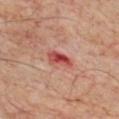Q: Where on the body is the lesion?
A: the chest
Q: Illumination type?
A: cross-polarized
Q: What are the patient's age and sex?
A: male, roughly 70 years of age
Q: What kind of image is this?
A: ~15 mm tile from a whole-body skin photo
Q: What did automated image analysis measure?
A: a footprint of about 5 mm² and a shape-asymmetry score of about 0.35 (0 = symmetric); a mean CIELAB color near L≈48 a*≈33 b*≈28, about 13 CIELAB-L* units darker than the surrounding skin, and a normalized lesion–skin contrast near 9; an automated nevus-likeness rating near 0 out of 100